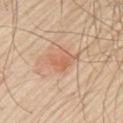diameter=≈3 mm | automated metrics=a lesion area of about 4.5 mm² and a shape eccentricity near 0.8; a lesion color around L≈62 a*≈23 b*≈34 in CIELAB and a normalized lesion–skin contrast near 5.5; a border-irregularity index near 4.5/10 and peripheral color asymmetry of about 0.5; a classifier nevus-likeness of about 90/100 | body site=the left upper arm | tile lighting=white-light illumination | image=~15 mm crop, total-body skin-cancer survey | subject=male, aged around 70.